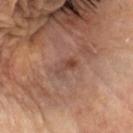notes: no biopsy performed (imaged during a skin exam) | image: total-body-photography crop, ~15 mm field of view | subject: female, approximately 65 years of age | site: the head or neck.